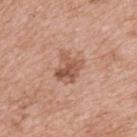Captured during whole-body skin photography for melanoma surveillance; the lesion was not biopsied.
From the upper back.
A male patient roughly 75 years of age.
The total-body-photography lesion software estimated a lesion area of about 7.5 mm², a shape eccentricity near 0.5, and two-axis asymmetry of about 0.25. And it measured a lesion color around L≈55 a*≈22 b*≈30 in CIELAB, a lesion–skin lightness drop of about 11, and a normalized border contrast of about 7.5. It also reported internal color variation of about 5.5 on a 0–10 scale and a peripheral color-asymmetry measure near 2.
Imaged with white-light lighting.
Cropped from a whole-body photographic skin survey; the tile spans about 15 mm.
Measured at roughly 3.5 mm in maximum diameter.